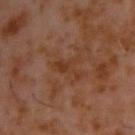notes: no biopsy performed (imaged during a skin exam)
body site: the back
imaging modality: 15 mm crop, total-body photography
patient: male, aged around 60
size: about 4 mm
automated lesion analysis: a footprint of about 4.5 mm², an eccentricity of roughly 0.9, and a shape-asymmetry score of about 0.6 (0 = symmetric); a mean CIELAB color near L≈33 a*≈20 b*≈29; a border-irregularity rating of about 7.5/10, internal color variation of about 1 on a 0–10 scale, and peripheral color asymmetry of about 0